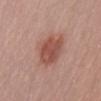This lesion was catalogued during total-body skin photography and was not selected for biopsy.
The patient is a male about 50 years old.
The recorded lesion diameter is about 5.5 mm.
The lesion is located on the abdomen.
This image is a 15 mm lesion crop taken from a total-body photograph.